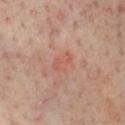Assessment:
Imaged during a routine full-body skin examination; the lesion was not biopsied and no histopathology is available.
Context:
The recorded lesion diameter is about 3 mm. The subject is a male aged 63–67. Automated image analysis of the tile measured a lesion area of about 4 mm², an eccentricity of roughly 0.85, and two-axis asymmetry of about 0.3. It also reported an average lesion color of about L≈53 a*≈25 b*≈28 (CIELAB) and a normalized border contrast of about 4.5. And it measured a border-irregularity rating of about 3.5/10, a within-lesion color-variation index near 2/10, and peripheral color asymmetry of about 0.5. Imaged with cross-polarized lighting. The lesion is located on the chest. Cropped from a whole-body photographic skin survey; the tile spans about 15 mm.